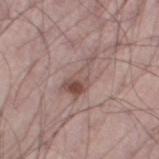location: the left thigh | lighting: white-light | acquisition: ~15 mm tile from a whole-body skin photo | patient: male, roughly 55 years of age.